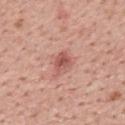The lesion was photographed on a routine skin check and not biopsied; there is no pathology result. Captured under white-light illumination. A 15 mm close-up tile from a total-body photography series done for melanoma screening. The lesion is located on the upper back. The lesion's longest dimension is about 2.5 mm. The lesion-visualizer software estimated an outline eccentricity of about 0.65 (0 = round, 1 = elongated) and a shape-asymmetry score of about 0.3 (0 = symmetric). The software also gave a mean CIELAB color near L≈55 a*≈28 b*≈26 and a normalized lesion–skin contrast near 7.5. And it measured a detector confidence of about 100 out of 100 that the crop contains a lesion. A male patient, about 60 years old.